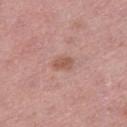No biopsy was performed on this lesion — it was imaged during a full skin examination and was not determined to be concerning.
The lesion is located on the right thigh.
The subject is a female aged approximately 45.
Captured under white-light illumination.
A region of skin cropped from a whole-body photographic capture, roughly 15 mm wide.